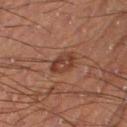No biopsy was performed on this lesion — it was imaged during a full skin examination and was not determined to be concerning. Automated image analysis of the tile measured an eccentricity of roughly 0.8. And it measured a border-irregularity rating of about 1.5/10, a color-variation rating of about 3.5/10, and peripheral color asymmetry of about 1. It also reported a detector confidence of about 100 out of 100 that the crop contains a lesion. The lesion is located on the leg. Cropped from a whole-body photographic skin survey; the tile spans about 15 mm. A male patient, in their mid- to late 50s. Imaged with cross-polarized lighting.